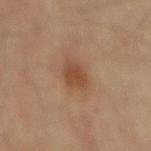Impression: Captured during whole-body skin photography for melanoma surveillance; the lesion was not biopsied. Background: The subject is a male aged 83 or older. A roughly 15 mm field-of-view crop from a total-body skin photograph. The recorded lesion diameter is about 3.5 mm. The lesion is on the mid back.